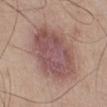Case summary:
* biopsy status: no biopsy performed (imaged during a skin exam)
* image-analysis metrics: a shape eccentricity near 0.7
* image source: 15 mm crop, total-body photography
* tile lighting: white-light illumination
* patient: male, aged approximately 45
* diameter: ~7.5 mm (longest diameter)
* body site: the leg